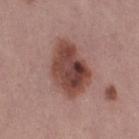This lesion was catalogued during total-body skin photography and was not selected for biopsy.
The patient is a female aged around 50.
A lesion tile, about 15 mm wide, cut from a 3D total-body photograph.
From the leg.